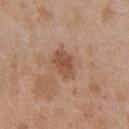Q: Is there a histopathology result?
A: no biopsy performed (imaged during a skin exam)
Q: Illumination type?
A: white-light
Q: Where on the body is the lesion?
A: the chest
Q: Patient demographics?
A: female, approximately 40 years of age
Q: Lesion size?
A: ~3.5 mm (longest diameter)
Q: What did automated image analysis measure?
A: a footprint of about 7 mm², a shape eccentricity near 0.75, and a shape-asymmetry score of about 0.2 (0 = symmetric); an automated nevus-likeness rating near 5 out of 100 and lesion-presence confidence of about 100/100
Q: What kind of image is this?
A: ~15 mm tile from a whole-body skin photo